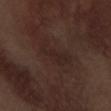<case>
  <biopsy_status>not biopsied; imaged during a skin examination</biopsy_status>
  <site>left forearm</site>
  <image>
    <source>total-body photography crop</source>
    <field_of_view_mm>15</field_of_view_mm>
  </image>
  <lighting>white-light</lighting>
  <lesion_size>
    <long_diameter_mm_approx>4.5</long_diameter_mm_approx>
  </lesion_size>
  <patient>
    <sex>male</sex>
    <age_approx>70</age_approx>
  </patient>
  <automated_metrics>
    <cielab_L>23</cielab_L>
    <cielab_a>15</cielab_a>
    <cielab_b>18</cielab_b>
    <vs_skin_darker_L>4.0</vs_skin_darker_L>
    <vs_skin_contrast_norm>5.0</vs_skin_contrast_norm>
    <nevus_likeness_0_100>0</nevus_likeness_0_100>
    <lesion_detection_confidence_0_100>100</lesion_detection_confidence_0_100>
  </automated_metrics>
</case>